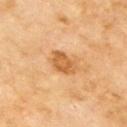Q: Is there a histopathology result?
A: no biopsy performed (imaged during a skin exam)
Q: Patient demographics?
A: male, aged around 70
Q: What kind of image is this?
A: total-body-photography crop, ~15 mm field of view
Q: Lesion location?
A: the right upper arm
Q: Illumination type?
A: cross-polarized
Q: What did automated image analysis measure?
A: a lesion color around L≈59 a*≈24 b*≈44 in CIELAB, a lesion–skin lightness drop of about 11, and a lesion-to-skin contrast of about 8 (normalized; higher = more distinct); a border-irregularity index near 2.5/10 and peripheral color asymmetry of about 0.5; a classifier nevus-likeness of about 55/100 and a detector confidence of about 100 out of 100 that the crop contains a lesion